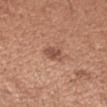biopsy_status: not biopsied; imaged during a skin examination
lesion_size:
  long_diameter_mm_approx: 2.5
image:
  source: total-body photography crop
  field_of_view_mm: 15
patient:
  sex: female
  age_approx: 30
lighting: white-light
site: right forearm